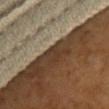Captured during whole-body skin photography for melanoma surveillance; the lesion was not biopsied.
A female subject, in their mid-50s.
Measured at roughly 2.5 mm in maximum diameter.
On the chest.
A lesion tile, about 15 mm wide, cut from a 3D total-body photograph.
Automated tile analysis of the lesion measured a border-irregularity index near 3.5/10, internal color variation of about 2 on a 0–10 scale, and radial color variation of about 0.5. And it measured a lesion-detection confidence of about 0/100.